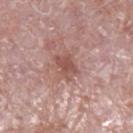The lesion was tiled from a total-body skin photograph and was not biopsied.
Automated tile analysis of the lesion measured a border-irregularity rating of about 3/10, a within-lesion color-variation index near 2.5/10, and radial color variation of about 1.
From the left lower leg.
About 3 mm across.
A male patient aged around 75.
The tile uses white-light illumination.
A roughly 15 mm field-of-view crop from a total-body skin photograph.